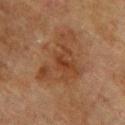<lesion>
  <biopsy_status>not biopsied; imaged during a skin examination</biopsy_status>
  <image>
    <source>total-body photography crop</source>
    <field_of_view_mm>15</field_of_view_mm>
  </image>
  <patient>
    <sex>male</sex>
    <age_approx>75</age_approx>
  </patient>
  <automated_metrics>
    <eccentricity>0.65</eccentricity>
    <shape_asymmetry>0.65</shape_asymmetry>
    <border_irregularity_0_10>9.0</border_irregularity_0_10>
    <color_variation_0_10>4.5</color_variation_0_10>
    <peripheral_color_asymmetry>1.5</peripheral_color_asymmetry>
    <lesion_detection_confidence_0_100>100</lesion_detection_confidence_0_100>
  </automated_metrics>
  <site>upper back</site>
  <lighting>cross-polarized</lighting>
</lesion>